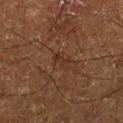Clinical impression:
Captured during whole-body skin photography for melanoma surveillance; the lesion was not biopsied.
Acquisition and patient details:
Longest diameter approximately 3 mm. The total-body-photography lesion software estimated a footprint of about 3 mm² and a symmetry-axis asymmetry near 0.4. The software also gave a within-lesion color-variation index near 0/10 and radial color variation of about 0. The analysis additionally found an automated nevus-likeness rating near 0 out of 100 and a detector confidence of about 85 out of 100 that the crop contains a lesion. Imaged with cross-polarized lighting. A male subject, in their mid-60s. This image is a 15 mm lesion crop taken from a total-body photograph. Located on the right lower leg.